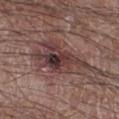This lesion was catalogued during total-body skin photography and was not selected for biopsy. Longest diameter approximately 10 mm. Located on the right lower leg. A 15 mm crop from a total-body photograph taken for skin-cancer surveillance. A male subject approximately 65 years of age. The tile uses white-light illumination.